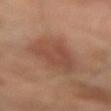Q: Is there a histopathology result?
A: imaged on a skin check; not biopsied
Q: Lesion size?
A: ~7 mm (longest diameter)
Q: How was the tile lit?
A: cross-polarized illumination
Q: What are the patient's age and sex?
A: male, aged approximately 65
Q: How was this image acquired?
A: ~15 mm crop, total-body skin-cancer survey
Q: What is the anatomic site?
A: the left forearm